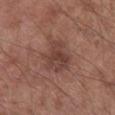* follow-up: no biopsy performed (imaged during a skin exam)
* illumination: white-light illumination
* body site: the right lower leg
* image-analysis metrics: a footprint of about 10 mm², an eccentricity of roughly 0.55, and a shape-asymmetry score of about 0.35 (0 = symmetric); peripheral color asymmetry of about 1.5; a nevus-likeness score of about 10/100 and lesion-presence confidence of about 100/100
* patient: male, in their mid-50s
* imaging modality: 15 mm crop, total-body photography
* diameter: ~4.5 mm (longest diameter)